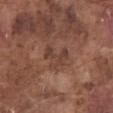patient:
  sex: male
  age_approx: 75
image:
  source: total-body photography crop
  field_of_view_mm: 15
lighting: white-light
site: front of the torso
lesion_size:
  long_diameter_mm_approx: 3.5